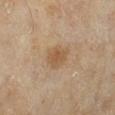Context: A 15 mm close-up extracted from a 3D total-body photography capture. The lesion is on the left lower leg. A female patient aged approximately 60. Imaged with cross-polarized lighting.